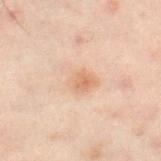Case summary:
* follow-up: no biopsy performed (imaged during a skin exam)
* body site: the left leg
* size: ≈4.5 mm
* patient: male, aged 48–52
* illumination: cross-polarized illumination
* image source: ~15 mm crop, total-body skin-cancer survey